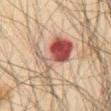Q: Who is the patient?
A: male, about 65 years old
Q: What is the anatomic site?
A: the chest
Q: How was this image acquired?
A: total-body-photography crop, ~15 mm field of view
Q: How large is the lesion?
A: about 6.5 mm